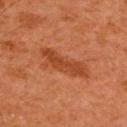biopsy status: catalogued during a skin exam; not biopsied | lesion diameter: ~6.5 mm (longest diameter) | automated metrics: about 9 CIELAB-L* units darker than the surrounding skin and a normalized border contrast of about 7; an automated nevus-likeness rating near 5 out of 100 | body site: the upper back | illumination: cross-polarized illumination | subject: male, approximately 65 years of age | image source: ~15 mm crop, total-body skin-cancer survey.